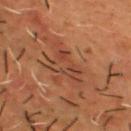<tbp_lesion>
  <biopsy_status>not biopsied; imaged during a skin examination</biopsy_status>
  <site>chest</site>
  <lesion_size>
    <long_diameter_mm_approx>5.5</long_diameter_mm_approx>
  </lesion_size>
  <patient>
    <sex>male</sex>
    <age_approx>50</age_approx>
  </patient>
  <automated_metrics>
    <area_mm2_approx>9.5</area_mm2_approx>
    <shape_asymmetry>0.55</shape_asymmetry>
    <color_variation_0_10>5.0</color_variation_0_10>
    <peripheral_color_asymmetry>1.5</peripheral_color_asymmetry>
  </automated_metrics>
  <image>
    <source>total-body photography crop</source>
    <field_of_view_mm>15</field_of_view_mm>
  </image>
  <lighting>cross-polarized</lighting>
</tbp_lesion>